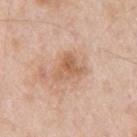A male subject aged around 55. Automated image analysis of the tile measured a mean CIELAB color near L≈61 a*≈21 b*≈33 and a lesion–skin lightness drop of about 10. The software also gave border irregularity of about 4.5 on a 0–10 scale, a color-variation rating of about 5/10, and peripheral color asymmetry of about 2. Located on the arm. Longest diameter approximately 4 mm. A 15 mm crop from a total-body photograph taken for skin-cancer surveillance. The tile uses white-light illumination.